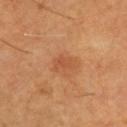The lesion is on the front of the torso. A male subject, approximately 60 years of age. The lesion-visualizer software estimated an average lesion color of about L≈49 a*≈25 b*≈36 (CIELAB), about 7 CIELAB-L* units darker than the surrounding skin, and a normalized lesion–skin contrast near 5. And it measured a classifier nevus-likeness of about 5/100 and lesion-presence confidence of about 100/100. A 15 mm close-up tile from a total-body photography series done for melanoma screening. Longest diameter approximately 2.5 mm. This is a cross-polarized tile.